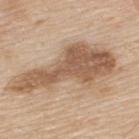<tbp_lesion>
<lesion_size>
  <long_diameter_mm_approx>12.5</long_diameter_mm_approx>
</lesion_size>
<patient>
  <sex>male</sex>
  <age_approx>80</age_approx>
</patient>
<site>upper back</site>
<lighting>white-light</lighting>
<image>
  <source>total-body photography crop</source>
  <field_of_view_mm>15</field_of_view_mm>
</image>
</tbp_lesion>